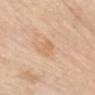{"biopsy_status": "not biopsied; imaged during a skin examination", "site": "chest", "image": {"source": "total-body photography crop", "field_of_view_mm": 15}, "patient": {"sex": "female", "age_approx": 65}, "lesion_size": {"long_diameter_mm_approx": 2.5}, "lighting": "white-light"}